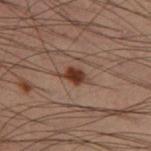Recorded during total-body skin imaging; not selected for excision or biopsy.
A lesion tile, about 15 mm wide, cut from a 3D total-body photograph.
The subject is a male in their mid- to late 50s.
Imaged with cross-polarized lighting.
From the leg.
An algorithmic analysis of the crop reported a footprint of about 4 mm², a shape eccentricity near 0.75, and a symmetry-axis asymmetry near 0.3. The software also gave a border-irregularity rating of about 2.5/10, internal color variation of about 2.5 on a 0–10 scale, and peripheral color asymmetry of about 1.